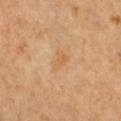follow-up = imaged on a skin check; not biopsied | body site = the chest | lesion size = about 2.5 mm | image = total-body-photography crop, ~15 mm field of view | subject = female, aged approximately 70 | tile lighting = cross-polarized.